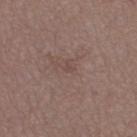workup — catalogued during a skin exam; not biopsied | size — ≈1 mm | lighting — white-light illumination | body site — the leg | imaging modality — ~15 mm tile from a whole-body skin photo | subject — female, aged around 30.